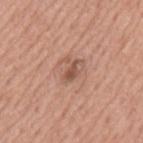The lesion was tiled from a total-body skin photograph and was not biopsied.
A close-up tile cropped from a whole-body skin photograph, about 15 mm across.
The subject is a male aged approximately 75.
Imaged with white-light lighting.
The lesion-visualizer software estimated a lesion color around L≈52 a*≈21 b*≈28 in CIELAB, roughly 11 lightness units darker than nearby skin, and a normalized lesion–skin contrast near 7.5. The analysis additionally found a border-irregularity rating of about 3.5/10 and internal color variation of about 0.5 on a 0–10 scale. The analysis additionally found a nevus-likeness score of about 5/100.
About 2.5 mm across.
From the mid back.